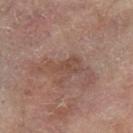Captured during whole-body skin photography for melanoma surveillance; the lesion was not biopsied.